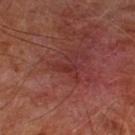biopsy_status: not biopsied; imaged during a skin examination
patient:
  sex: male
  age_approx: 65
site: leg
automated_metrics:
  area_mm2_approx: 2.5
  eccentricity: 0.9
  shape_asymmetry: 0.4
image:
  source: total-body photography crop
  field_of_view_mm: 15